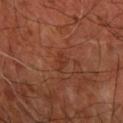Clinical impression:
This lesion was catalogued during total-body skin photography and was not selected for biopsy.
Background:
The subject is aged 63–67. A 15 mm crop from a total-body photograph taken for skin-cancer surveillance. Automated tile analysis of the lesion measured a lesion area of about 3 mm², an eccentricity of roughly 0.8, and a symmetry-axis asymmetry near 0.4. And it measured a lesion color around L≈34 a*≈26 b*≈30 in CIELAB, about 5 CIELAB-L* units darker than the surrounding skin, and a normalized border contrast of about 4.5. The software also gave a border-irregularity index near 4/10 and peripheral color asymmetry of about 0.5. Measured at roughly 2.5 mm in maximum diameter. The lesion is on the right forearm.